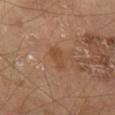Recorded during total-body skin imaging; not selected for excision or biopsy. A male patient in their 70s. This image is a 15 mm lesion crop taken from a total-body photograph. The tile uses cross-polarized illumination. The recorded lesion diameter is about 3 mm. On the right thigh.